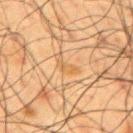Recorded during total-body skin imaging; not selected for excision or biopsy. A male patient, about 60 years old. About 3 mm across. The lesion is on the upper back. A 15 mm crop from a total-body photograph taken for skin-cancer surveillance.